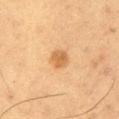<lesion>
<biopsy_status>not biopsied; imaged during a skin examination</biopsy_status>
<site>left thigh</site>
<image>
  <source>total-body photography crop</source>
  <field_of_view_mm>15</field_of_view_mm>
</image>
<lighting>cross-polarized</lighting>
<patient>
  <sex>male</sex>
  <age_approx>55</age_approx>
</patient>
<lesion_size>
  <long_diameter_mm_approx>3.0</long_diameter_mm_approx>
</lesion_size>
</lesion>